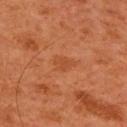| feature | finding |
|---|---|
| biopsy status | no biopsy performed (imaged during a skin exam) |
| location | the back |
| patient | male, about 65 years old |
| automated metrics | a footprint of about 3.5 mm², a shape eccentricity near 0.75, and a shape-asymmetry score of about 0.35 (0 = symmetric); an average lesion color of about L≈46 a*≈28 b*≈38 (CIELAB), a lesion–skin lightness drop of about 6, and a lesion-to-skin contrast of about 4.5 (normalized; higher = more distinct); a border-irregularity index near 3/10 and a peripheral color-asymmetry measure near 0.5; a nevus-likeness score of about 10/100 and a lesion-detection confidence of about 100/100 |
| acquisition | ~15 mm tile from a whole-body skin photo |
| lighting | cross-polarized illumination |
| lesion diameter | ≈2.5 mm |